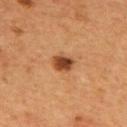{"image": {"source": "total-body photography crop", "field_of_view_mm": 15}, "patient": {"sex": "male", "age_approx": 55}, "site": "upper back"}